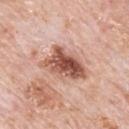Part of a total-body skin-imaging series; this lesion was reviewed on a skin check and was not flagged for biopsy. A male subject, in their 80s. The lesion's longest dimension is about 5.5 mm. A close-up tile cropped from a whole-body skin photograph, about 15 mm across. Located on the back. This is a white-light tile.